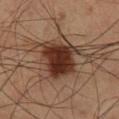Context: Measured at roughly 5.5 mm in maximum diameter. The patient is a male aged 58–62. A 15 mm close-up tile from a total-body photography series done for melanoma screening. Located on the right lower leg. Captured under cross-polarized illumination.